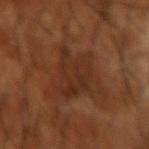Imaged during a routine full-body skin examination; the lesion was not biopsied and no histopathology is available. The recorded lesion diameter is about 5 mm. This is a cross-polarized tile. The patient is a male roughly 65 years of age. The lesion-visualizer software estimated a lesion area of about 15 mm², a shape eccentricity near 0.45, and two-axis asymmetry of about 0.4. The analysis additionally found a border-irregularity index near 4.5/10 and a color-variation rating of about 3.5/10. The software also gave an automated nevus-likeness rating near 0 out of 100 and a detector confidence of about 95 out of 100 that the crop contains a lesion. Located on the right forearm. A close-up tile cropped from a whole-body skin photograph, about 15 mm across.